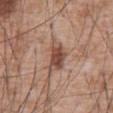<tbp_lesion>
  <biopsy_status>not biopsied; imaged during a skin examination</biopsy_status>
  <lesion_size>
    <long_diameter_mm_approx>4.0</long_diameter_mm_approx>
  </lesion_size>
  <site>abdomen</site>
  <patient>
    <sex>male</sex>
    <age_approx>60</age_approx>
  </patient>
  <image>
    <source>total-body photography crop</source>
    <field_of_view_mm>15</field_of_view_mm>
  </image>
  <lighting>white-light</lighting>
</tbp_lesion>